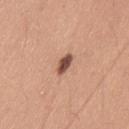Captured during whole-body skin photography for melanoma surveillance; the lesion was not biopsied. A female patient, about 45 years old. The tile uses white-light illumination. A roughly 15 mm field-of-view crop from a total-body skin photograph. The lesion is located on the left thigh.